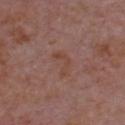Case summary:
• follow-up · no biopsy performed (imaged during a skin exam)
• lesion size · about 3 mm
• imaging modality · 15 mm crop, total-body photography
• illumination · white-light illumination
• automated lesion analysis · border irregularity of about 7.5 on a 0–10 scale, internal color variation of about 0 on a 0–10 scale, and a peripheral color-asymmetry measure near 0; a detector confidence of about 100 out of 100 that the crop contains a lesion
• patient · male, aged around 65
• body site · the chest